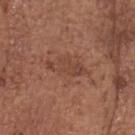Case summary:
- notes — imaged on a skin check; not biopsied
- location — the head or neck
- TBP lesion metrics — a normalized lesion–skin contrast near 5.5; a nevus-likeness score of about 5/100 and lesion-presence confidence of about 100/100
- illumination — white-light
- patient — male, roughly 75 years of age
- lesion size — ≈5 mm
- imaging modality — ~15 mm tile from a whole-body skin photo